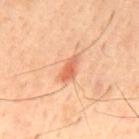- biopsy status — imaged on a skin check; not biopsied
- tile lighting — cross-polarized
- location — the back
- patient — male, approximately 45 years of age
- automated lesion analysis — a nevus-likeness score of about 45/100 and a detector confidence of about 100 out of 100 that the crop contains a lesion
- image source — total-body-photography crop, ~15 mm field of view
- lesion size — ≈3.5 mm